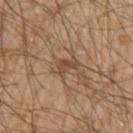The lesion was tiled from a total-body skin photograph and was not biopsied. The recorded lesion diameter is about 3 mm. The lesion-visualizer software estimated an average lesion color of about L≈44 a*≈17 b*≈30 (CIELAB), a lesion–skin lightness drop of about 8, and a normalized lesion–skin contrast near 6. The analysis additionally found border irregularity of about 5 on a 0–10 scale and internal color variation of about 2 on a 0–10 scale. The analysis additionally found a nevus-likeness score of about 0/100 and a detector confidence of about 75 out of 100 that the crop contains a lesion. Captured under cross-polarized illumination. A region of skin cropped from a whole-body photographic capture, roughly 15 mm wide. From the left upper arm. The subject is a male aged around 55.